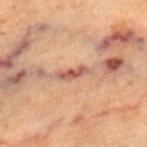Captured during whole-body skin photography for melanoma surveillance; the lesion was not biopsied.
A 15 mm close-up extracted from a 3D total-body photography capture.
From the right lower leg.
A female subject aged 68–72.
About 4 mm across.